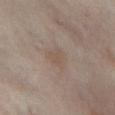This lesion was catalogued during total-body skin photography and was not selected for biopsy. From the abdomen. A lesion tile, about 15 mm wide, cut from a 3D total-body photograph. The subject is a female in their mid- to late 50s.